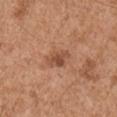This lesion was catalogued during total-body skin photography and was not selected for biopsy.
Located on the right upper arm.
A male subject, roughly 65 years of age.
This image is a 15 mm lesion crop taken from a total-body photograph.
Automated image analysis of the tile measured an eccentricity of roughly 0.85 and two-axis asymmetry of about 0.25. It also reported a lesion color around L≈50 a*≈23 b*≈32 in CIELAB and a normalized border contrast of about 7. It also reported border irregularity of about 2.5 on a 0–10 scale and internal color variation of about 2.5 on a 0–10 scale. And it measured an automated nevus-likeness rating near 30 out of 100 and lesion-presence confidence of about 100/100.
Longest diameter approximately 3.5 mm.
Imaged with white-light lighting.